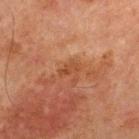Q: Was this lesion biopsied?
A: catalogued during a skin exam; not biopsied
Q: Who is the patient?
A: male, about 65 years old
Q: What is the imaging modality?
A: ~15 mm crop, total-body skin-cancer survey
Q: Lesion location?
A: the chest